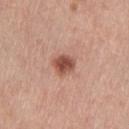Q: Was this lesion biopsied?
A: catalogued during a skin exam; not biopsied
Q: Lesion location?
A: the left thigh
Q: What lighting was used for the tile?
A: white-light
Q: How was this image acquired?
A: ~15 mm tile from a whole-body skin photo
Q: Lesion size?
A: ≈2.5 mm
Q: Patient demographics?
A: female, roughly 55 years of age